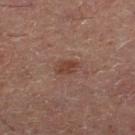{
  "biopsy_status": "not biopsied; imaged during a skin examination",
  "lighting": "cross-polarized",
  "image": {
    "source": "total-body photography crop",
    "field_of_view_mm": 15
  },
  "patient": {
    "sex": "male",
    "age_approx": 50
  },
  "site": "right lower leg"
}